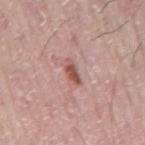No biopsy was performed on this lesion — it was imaged during a full skin examination and was not determined to be concerning. The subject is a male roughly 75 years of age. From the mid back. A 15 mm crop from a total-body photograph taken for skin-cancer surveillance. Captured under white-light illumination. Longest diameter approximately 3 mm.